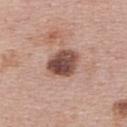This lesion was catalogued during total-body skin photography and was not selected for biopsy.
The tile uses white-light illumination.
This image is a 15 mm lesion crop taken from a total-body photograph.
The lesion is on the upper back.
The lesion's longest dimension is about 4 mm.
Automated tile analysis of the lesion measured an eccentricity of roughly 0.5 and two-axis asymmetry of about 0.15. It also reported a lesion–skin lightness drop of about 17 and a lesion-to-skin contrast of about 11.5 (normalized; higher = more distinct). It also reported border irregularity of about 1.5 on a 0–10 scale, internal color variation of about 6 on a 0–10 scale, and a peripheral color-asymmetry measure near 2.
A female subject aged 48 to 52.